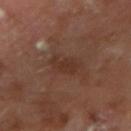Imaged during a routine full-body skin examination; the lesion was not biopsied and no histopathology is available.
A 15 mm close-up extracted from a 3D total-body photography capture.
The lesion's longest dimension is about 3 mm.
Located on the right upper arm.
The tile uses cross-polarized illumination.
The subject is a male aged around 65.